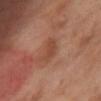Imaged during a routine full-body skin examination; the lesion was not biopsied and no histopathology is available.
The subject is a female approximately 55 years of age.
The tile uses cross-polarized illumination.
The lesion is located on the abdomen.
Cropped from a total-body skin-imaging series; the visible field is about 15 mm.
The total-body-photography lesion software estimated an automated nevus-likeness rating near 0 out of 100.